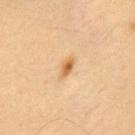Findings:
- workup — total-body-photography surveillance lesion; no biopsy
- lesion size — ≈3 mm
- subject — male, roughly 55 years of age
- image source — 15 mm crop, total-body photography
- location — the chest
- illumination — cross-polarized